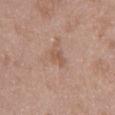Impression:
This lesion was catalogued during total-body skin photography and was not selected for biopsy.
Clinical summary:
The patient is a male about 50 years old. An algorithmic analysis of the crop reported a nevus-likeness score of about 0/100 and lesion-presence confidence of about 100/100. The lesion is located on the abdomen. Approximately 2.5 mm at its widest. Captured under white-light illumination. This image is a 15 mm lesion crop taken from a total-body photograph.